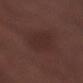<lesion>
<biopsy_status>not biopsied; imaged during a skin examination</biopsy_status>
<lighting>white-light</lighting>
<site>right forearm</site>
<image>
  <source>total-body photography crop</source>
  <field_of_view_mm>15</field_of_view_mm>
</image>
<patient>
  <sex>male</sex>
  <age_approx>50</age_approx>
</patient>
</lesion>